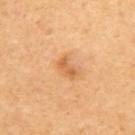- follow-up · catalogued during a skin exam; not biopsied
- patient · female, approximately 50 years of age
- tile lighting · cross-polarized
- site · the upper back
- automated metrics · a border-irregularity rating of about 3/10 and peripheral color asymmetry of about 0
- image source · 15 mm crop, total-body photography
- size · ~2.5 mm (longest diameter)